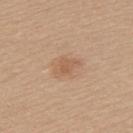From the back. Measured at roughly 3 mm in maximum diameter. Cropped from a total-body skin-imaging series; the visible field is about 15 mm. The subject is a female aged approximately 35. This is a white-light tile. An algorithmic analysis of the crop reported an eccentricity of roughly 0.75 and two-axis asymmetry of about 0.25. And it measured a normalized lesion–skin contrast near 5.5. The analysis additionally found a border-irregularity index near 2.5/10, a within-lesion color-variation index near 2/10, and peripheral color asymmetry of about 0.5.